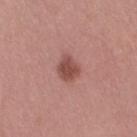Findings:
- workup — imaged on a skin check; not biopsied
- lesion diameter — about 2.5 mm
- anatomic site — the right thigh
- imaging modality — ~15 mm tile from a whole-body skin photo
- subject — female, about 30 years old